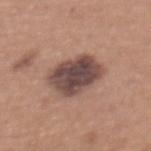{"lesion_size": {"long_diameter_mm_approx": 5.0}, "site": "upper back", "image": {"source": "total-body photography crop", "field_of_view_mm": 15}, "patient": {"sex": "female", "age_approx": 50}, "lighting": "white-light"}